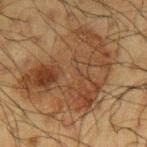Case summary:
* follow-up — imaged on a skin check; not biopsied